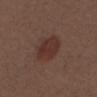Findings:
- notes · imaged on a skin check; not biopsied
- imaging modality · 15 mm crop, total-body photography
- patient · female, aged 38 to 42
- location · the head or neck
- diameter · ~4.5 mm (longest diameter)